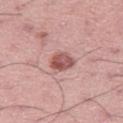Clinical impression:
The lesion was photographed on a routine skin check and not biopsied; there is no pathology result.
Context:
A lesion tile, about 15 mm wide, cut from a 3D total-body photograph. Located on the right thigh. This is a white-light tile. Longest diameter approximately 3 mm. A male subject, about 45 years old. An algorithmic analysis of the crop reported a lesion area of about 5.5 mm², a shape eccentricity near 0.6, and a symmetry-axis asymmetry near 0.2.